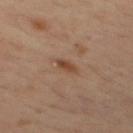biopsy status: imaged on a skin check; not biopsied
site: the mid back
lighting: cross-polarized
lesion diameter: ~2.5 mm (longest diameter)
patient: male, about 30 years old
acquisition: ~15 mm crop, total-body skin-cancer survey